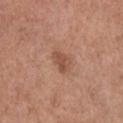Assessment:
Part of a total-body skin-imaging series; this lesion was reviewed on a skin check and was not flagged for biopsy.
Background:
A close-up tile cropped from a whole-body skin photograph, about 15 mm across. A female subject, in their mid-60s. The total-body-photography lesion software estimated a footprint of about 4.5 mm² and a symmetry-axis asymmetry near 0.25. And it measured a mean CIELAB color near L≈51 a*≈23 b*≈29 and a lesion-to-skin contrast of about 6.5 (normalized; higher = more distinct). And it measured a nevus-likeness score of about 45/100. Located on the chest. About 3 mm across.